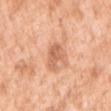Background: The subject is a female approximately 40 years of age. Imaged with white-light lighting. From the right upper arm. A 15 mm close-up tile from a total-body photography series done for melanoma screening. About 3.5 mm across.